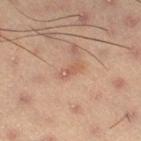Impression:
Captured during whole-body skin photography for melanoma surveillance; the lesion was not biopsied.